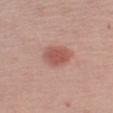{
  "biopsy_status": "not biopsied; imaged during a skin examination",
  "image": {
    "source": "total-body photography crop",
    "field_of_view_mm": 15
  },
  "lighting": "white-light",
  "patient": {
    "sex": "male",
    "age_approx": 60
  },
  "lesion_size": {
    "long_diameter_mm_approx": 4.0
  },
  "site": "left upper arm"
}